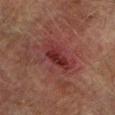  biopsy_status: not biopsied; imaged during a skin examination
  automated_metrics:
    cielab_L: 26
    cielab_a: 25
    cielab_b: 20
    vs_skin_darker_L: 8.0
    vs_skin_contrast_norm: 8.0
  patient:
    sex: male
    age_approx: 75
  image:
    source: total-body photography crop
    field_of_view_mm: 15
  site: left lower leg
  lesion_size:
    long_diameter_mm_approx: 4.5